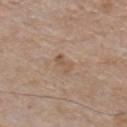Part of a total-body skin-imaging series; this lesion was reviewed on a skin check and was not flagged for biopsy. A male patient, aged approximately 55. The lesion is on the chest. A 15 mm close-up tile from a total-body photography series done for melanoma screening. The recorded lesion diameter is about 2.5 mm.